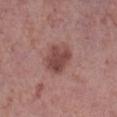biopsy status: total-body-photography surveillance lesion; no biopsy
subject: female, about 40 years old
acquisition: 15 mm crop, total-body photography
body site: the right lower leg
lighting: white-light illumination
size: ~4 mm (longest diameter)